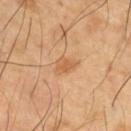This lesion was catalogued during total-body skin photography and was not selected for biopsy. This is a cross-polarized tile. The lesion is located on the right thigh. The patient is a male roughly 65 years of age. A roughly 15 mm field-of-view crop from a total-body skin photograph.